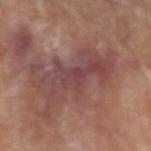No biopsy was performed on this lesion — it was imaged during a full skin examination and was not determined to be concerning. The lesion's longest dimension is about 9 mm. The tile uses white-light illumination. This image is a 15 mm lesion crop taken from a total-body photograph. The subject is a male aged 78 to 82. An algorithmic analysis of the crop reported a lesion area of about 22 mm² and an outline eccentricity of about 0.95 (0 = round, 1 = elongated). It also reported border irregularity of about 8 on a 0–10 scale, a within-lesion color-variation index near 5/10, and a peripheral color-asymmetry measure near 1.5. Located on the arm.